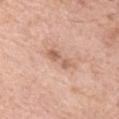The lesion was tiled from a total-body skin photograph and was not biopsied. A close-up tile cropped from a whole-body skin photograph, about 15 mm across. About 4 mm across. Located on the left upper arm. The subject is a male in their mid-50s. This is a white-light tile.